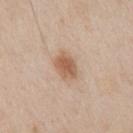Findings:
• follow-up — imaged on a skin check; not biopsied
• location — the front of the torso
• image source — ~15 mm tile from a whole-body skin photo
• subject — male, aged 58 to 62
• lighting — white-light